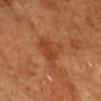Case summary:
– workup: total-body-photography surveillance lesion; no biopsy
– patient: male, approximately 55 years of age
– image source: ~15 mm tile from a whole-body skin photo
– lighting: cross-polarized illumination
– automated lesion analysis: a mean CIELAB color near L≈45 a*≈26 b*≈39, a lesion–skin lightness drop of about 7, and a lesion-to-skin contrast of about 6 (normalized; higher = more distinct); border irregularity of about 6 on a 0–10 scale, internal color variation of about 4 on a 0–10 scale, and a peripheral color-asymmetry measure near 1; an automated nevus-likeness rating near 0 out of 100 and a lesion-detection confidence of about 100/100
– body site: the head or neck